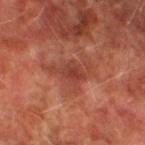Imaged during a routine full-body skin examination; the lesion was not biopsied and no histopathology is available. A 15 mm crop from a total-body photograph taken for skin-cancer surveillance. From the leg. The patient is a male roughly 75 years of age.